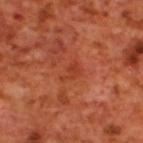| field | value |
|---|---|
| follow-up | total-body-photography surveillance lesion; no biopsy |
| body site | the upper back |
| subject | male, aged 68–72 |
| lighting | cross-polarized |
| automated lesion analysis | a shape eccentricity near 0.8 and two-axis asymmetry of about 0.55 |
| imaging modality | 15 mm crop, total-body photography |